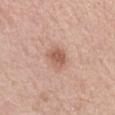– follow-up · catalogued during a skin exam; not biopsied
– image · ~15 mm crop, total-body skin-cancer survey
– body site · the left upper arm
– size · ≈2.5 mm
– subject · female, about 50 years old
– illumination · white-light